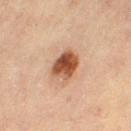| field | value |
|---|---|
| workup | catalogued during a skin exam; not biopsied |
| image-analysis metrics | a mean CIELAB color near L≈43 a*≈22 b*≈30 and a lesion–skin lightness drop of about 16; a border-irregularity index near 1.5/10, internal color variation of about 5.5 on a 0–10 scale, and radial color variation of about 2; a lesion-detection confidence of about 100/100 |
| subject | female, in their mid- to late 60s |
| illumination | cross-polarized illumination |
| diameter | about 3.5 mm |
| site | the right thigh |
| image | ~15 mm tile from a whole-body skin photo |